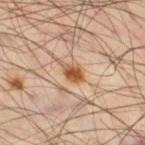The lesion was tiled from a total-body skin photograph and was not biopsied. The subject is a male approximately 50 years of age. Captured under cross-polarized illumination. A close-up tile cropped from a whole-body skin photograph, about 15 mm across. Longest diameter approximately 3.5 mm. The lesion-visualizer software estimated a shape eccentricity near 0.85 and a shape-asymmetry score of about 0.35 (0 = symmetric). It also reported a classifier nevus-likeness of about 100/100 and a lesion-detection confidence of about 100/100. On the right thigh.